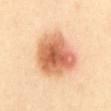  biopsy_status: not biopsied; imaged during a skin examination
  image:
    source: total-body photography crop
    field_of_view_mm: 15
  patient:
    sex: female
    age_approx: 60
  site: abdomen
  lesion_size:
    long_diameter_mm_approx: 6.0